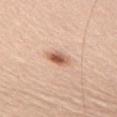{"biopsy_status": "not biopsied; imaged during a skin examination", "site": "right upper arm", "lesion_size": {"long_diameter_mm_approx": 3.0}, "automated_metrics": {"vs_skin_darker_L": 14.0, "vs_skin_contrast_norm": 9.0}, "patient": {"sex": "male", "age_approx": 35}, "image": {"source": "total-body photography crop", "field_of_view_mm": 15}, "lighting": "white-light"}